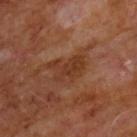Clinical impression: No biopsy was performed on this lesion — it was imaged during a full skin examination and was not determined to be concerning. Background: A male subject, about 60 years old. Imaged with cross-polarized lighting. Located on the chest. Cropped from a whole-body photographic skin survey; the tile spans about 15 mm. The lesion-visualizer software estimated a shape eccentricity near 0.75 and two-axis asymmetry of about 0.3. It also reported internal color variation of about 2 on a 0–10 scale and radial color variation of about 0.5. The analysis additionally found a classifier nevus-likeness of about 0/100 and a lesion-detection confidence of about 100/100. Approximately 4.5 mm at its widest.